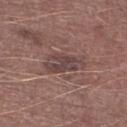Findings:
* TBP lesion metrics · a footprint of about 8.5 mm²; a lesion color around L≈42 a*≈17 b*≈18 in CIELAB, a lesion–skin lightness drop of about 9, and a normalized lesion–skin contrast near 8
* patient · male, aged approximately 75
* acquisition · ~15 mm crop, total-body skin-cancer survey
* tile lighting · white-light illumination
* lesion diameter · ≈5 mm
* location · the right lower leg